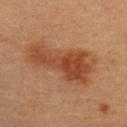Captured during whole-body skin photography for melanoma surveillance; the lesion was not biopsied.
The tile uses cross-polarized illumination.
The lesion-visualizer software estimated a footprint of about 27 mm², an outline eccentricity of about 0.9 (0 = round, 1 = elongated), and two-axis asymmetry of about 0.4. And it measured a lesion–skin lightness drop of about 9 and a normalized lesion–skin contrast near 7.5. And it measured a classifier nevus-likeness of about 70/100.
A lesion tile, about 15 mm wide, cut from a 3D total-body photograph.
About 8.5 mm across.
The lesion is on the upper back.
The subject is a female aged around 30.